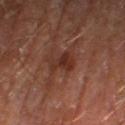Q: Was a biopsy performed?
A: imaged on a skin check; not biopsied
Q: What are the patient's age and sex?
A: male, aged 68–72
Q: What kind of image is this?
A: ~15 mm tile from a whole-body skin photo
Q: What is the anatomic site?
A: the right thigh